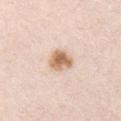The lesion was photographed on a routine skin check and not biopsied; there is no pathology result.
On the left upper arm.
A male patient in their mid-30s.
A region of skin cropped from a whole-body photographic capture, roughly 15 mm wide.